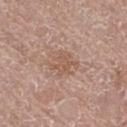* notes — imaged on a skin check; not biopsied
* site — the right thigh
* image-analysis metrics — a mean CIELAB color near L≈56 a*≈19 b*≈28, roughly 7 lightness units darker than nearby skin, and a normalized lesion–skin contrast near 5.5; internal color variation of about 2 on a 0–10 scale and peripheral color asymmetry of about 0.5; an automated nevus-likeness rating near 0 out of 100
* lesion diameter — ~3.5 mm (longest diameter)
* patient — female, roughly 75 years of age
* lighting — white-light
* acquisition — 15 mm crop, total-body photography